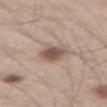Impression: The lesion was tiled from a total-body skin photograph and was not biopsied. Context: A male patient, aged approximately 60. Cropped from a whole-body photographic skin survey; the tile spans about 15 mm. The recorded lesion diameter is about 4 mm. From the right thigh.